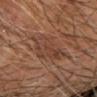notes — total-body-photography surveillance lesion; no biopsy | automated lesion analysis — an eccentricity of roughly 0.85 and a symmetry-axis asymmetry near 0.3; a lesion color around L≈41 a*≈21 b*≈28 in CIELAB, a lesion–skin lightness drop of about 7, and a normalized border contrast of about 5.5 | acquisition — total-body-photography crop, ~15 mm field of view | patient — male, about 65 years old | anatomic site — the right forearm | lighting — cross-polarized.